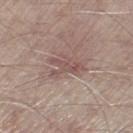Impression:
Part of a total-body skin-imaging series; this lesion was reviewed on a skin check and was not flagged for biopsy.
Clinical summary:
Measured at roughly 4 mm in maximum diameter. On the right lower leg. The tile uses white-light illumination. The patient is a male aged 63 to 67. A roughly 15 mm field-of-view crop from a total-body skin photograph.